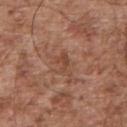workup: no biopsy performed (imaged during a skin exam) | tile lighting: white-light | TBP lesion metrics: a lesion–skin lightness drop of about 7 and a normalized lesion–skin contrast near 5.5; border irregularity of about 6.5 on a 0–10 scale and peripheral color asymmetry of about 0; a nevus-likeness score of about 0/100 and lesion-presence confidence of about 100/100 | lesion size: ~3 mm (longest diameter) | subject: male, aged 53–57 | body site: the back | acquisition: total-body-photography crop, ~15 mm field of view.